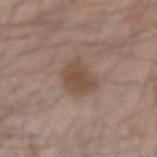The lesion is located on the left forearm.
A male patient, approximately 45 years of age.
The lesion's longest dimension is about 3.5 mm.
The tile uses white-light illumination.
An algorithmic analysis of the crop reported a mean CIELAB color near L≈48 a*≈15 b*≈25, about 9 CIELAB-L* units darker than the surrounding skin, and a normalized border contrast of about 7. The analysis additionally found a nevus-likeness score of about 50/100 and a detector confidence of about 100 out of 100 that the crop contains a lesion.
A lesion tile, about 15 mm wide, cut from a 3D total-body photograph.